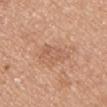{
  "biopsy_status": "not biopsied; imaged during a skin examination",
  "automated_metrics": {
    "shape_asymmetry": 0.3,
    "cielab_L": 59,
    "cielab_a": 22,
    "cielab_b": 32,
    "vs_skin_darker_L": 7.0,
    "vs_skin_contrast_norm": 5.0
  },
  "site": "left upper arm",
  "image": {
    "source": "total-body photography crop",
    "field_of_view_mm": 15
  },
  "patient": {
    "sex": "female",
    "age_approx": 35
  }
}